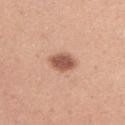<record>
<biopsy_status>not biopsied; imaged during a skin examination</biopsy_status>
<patient>
  <sex>female</sex>
  <age_approx>30</age_approx>
</patient>
<site>arm</site>
<image>
  <source>total-body photography crop</source>
  <field_of_view_mm>15</field_of_view_mm>
</image>
<lighting>white-light</lighting>
</record>